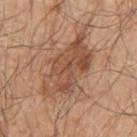This lesion was catalogued during total-body skin photography and was not selected for biopsy.
A male patient aged around 80.
The lesion is on the right upper arm.
This is a white-light tile.
Approximately 8 mm at its widest.
A lesion tile, about 15 mm wide, cut from a 3D total-body photograph.
Automated image analysis of the tile measured a nevus-likeness score of about 30/100 and a detector confidence of about 100 out of 100 that the crop contains a lesion.